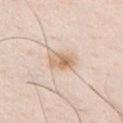Q: What is the imaging modality?
A: ~15 mm crop, total-body skin-cancer survey
Q: Patient demographics?
A: male, about 35 years old
Q: Lesion location?
A: the chest